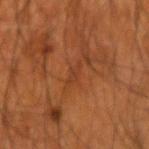biopsy status: no biopsy performed (imaged during a skin exam) | subject: male, roughly 55 years of age | image source: total-body-photography crop, ~15 mm field of view | body site: the right forearm | illumination: cross-polarized illumination | diameter: ~2.5 mm (longest diameter).